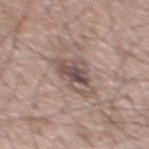The lesion-visualizer software estimated an eccentricity of roughly 0.75 and two-axis asymmetry of about 0.35. The analysis additionally found a border-irregularity index near 4/10 and internal color variation of about 7 on a 0–10 scale. And it measured a classifier nevus-likeness of about 0/100 and a lesion-detection confidence of about 90/100.
A lesion tile, about 15 mm wide, cut from a 3D total-body photograph.
A male subject, aged 63 to 67.
From the chest.
Longest diameter approximately 4.5 mm.
Captured under white-light illumination.